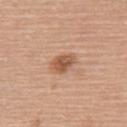follow-up: no biopsy performed (imaged during a skin exam) | site: the back | image: ~15 mm crop, total-body skin-cancer survey | size: about 3.5 mm | subject: female, in their mid-60s | tile lighting: white-light illumination.